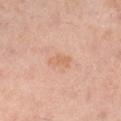Impression: Part of a total-body skin-imaging series; this lesion was reviewed on a skin check and was not flagged for biopsy. Context: Located on the leg. A female subject aged 38 to 42. The lesion's longest dimension is about 3 mm. A region of skin cropped from a whole-body photographic capture, roughly 15 mm wide. The tile uses cross-polarized illumination.